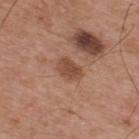| feature | finding |
|---|---|
| image source | total-body-photography crop, ~15 mm field of view |
| subject | male, in their mid- to late 50s |
| anatomic site | the upper back |